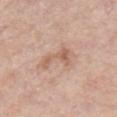biopsy status: total-body-photography surveillance lesion; no biopsy
size: ~4.5 mm (longest diameter)
image source: ~15 mm tile from a whole-body skin photo
subject: male, approximately 50 years of age
anatomic site: the chest
illumination: white-light